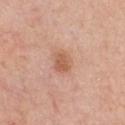workup: catalogued during a skin exam; not biopsied
acquisition: 15 mm crop, total-body photography
patient: female, roughly 55 years of age
site: the chest
tile lighting: white-light
automated lesion analysis: an area of roughly 5 mm², an eccentricity of roughly 0.65, and two-axis asymmetry of about 0.15
lesion diameter: ~2.5 mm (longest diameter)